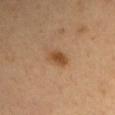automated metrics=a mean CIELAB color near L≈46 a*≈20 b*≈35, a lesion–skin lightness drop of about 10, and a normalized lesion–skin contrast near 8; a border-irregularity index near 2/10 and peripheral color asymmetry of about 0.5; a nevus-likeness score of about 95/100 and a detector confidence of about 100 out of 100 that the crop contains a lesion | image=~15 mm tile from a whole-body skin photo | subject=male, aged 53 to 57 | location=the arm | size=about 3 mm.